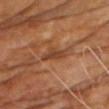| feature | finding |
|---|---|
| notes | total-body-photography surveillance lesion; no biopsy |
| image-analysis metrics | an area of roughly 5 mm², an outline eccentricity of about 0.85 (0 = round, 1 = elongated), and two-axis asymmetry of about 0.5; a mean CIELAB color near L≈42 a*≈23 b*≈33, about 9 CIELAB-L* units darker than the surrounding skin, and a normalized border contrast of about 7; a within-lesion color-variation index near 2/10 and radial color variation of about 0.5 |
| diameter | about 3.5 mm |
| illumination | cross-polarized |
| location | the chest |
| subject | male, about 70 years old |
| acquisition | total-body-photography crop, ~15 mm field of view |